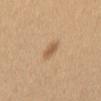<case>
  <biopsy_status>not biopsied; imaged during a skin examination</biopsy_status>
  <site>back</site>
  <image>
    <source>total-body photography crop</source>
    <field_of_view_mm>15</field_of_view_mm>
  </image>
  <lesion_size>
    <long_diameter_mm_approx>2.5</long_diameter_mm_approx>
  </lesion_size>
  <automated_metrics>
    <border_irregularity_0_10>2.0</border_irregularity_0_10>
    <peripheral_color_asymmetry>0.5</peripheral_color_asymmetry>
    <lesion_detection_confidence_0_100>100</lesion_detection_confidence_0_100>
  </automated_metrics>
  <patient>
    <sex>female</sex>
    <age_approx>60</age_approx>
  </patient>
  <lighting>white-light</lighting>
</case>